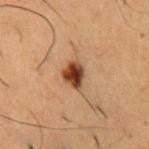This lesion was catalogued during total-body skin photography and was not selected for biopsy.